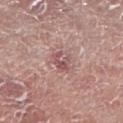Part of a total-body skin-imaging series; this lesion was reviewed on a skin check and was not flagged for biopsy. The lesion is on the right lower leg. This is a white-light tile. An algorithmic analysis of the crop reported a lesion color around L≈54 a*≈23 b*≈19 in CIELAB, roughly 9 lightness units darker than nearby skin, and a normalized lesion–skin contrast near 6.5. The software also gave a border-irregularity rating of about 3/10, a color-variation rating of about 7/10, and radial color variation of about 2.5. The software also gave a classifier nevus-likeness of about 0/100 and a lesion-detection confidence of about 95/100. A 15 mm crop from a total-body photograph taken for skin-cancer surveillance. A male subject, approximately 80 years of age. About 2.5 mm across.